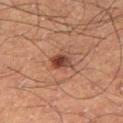Q: Is there a histopathology result?
A: imaged on a skin check; not biopsied